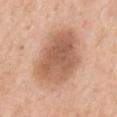Q: Was a biopsy performed?
A: catalogued during a skin exam; not biopsied
Q: What kind of image is this?
A: ~15 mm crop, total-body skin-cancer survey
Q: What are the patient's age and sex?
A: female, approximately 40 years of age
Q: What is the anatomic site?
A: the back